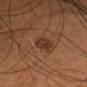Q: Was a biopsy performed?
A: total-body-photography surveillance lesion; no biopsy
Q: Patient demographics?
A: female, approximately 40 years of age
Q: What did automated image analysis measure?
A: a footprint of about 4.5 mm², an eccentricity of roughly 0.85, and a shape-asymmetry score of about 0.25 (0 = symmetric); an average lesion color of about L≈34 a*≈21 b*≈29 (CIELAB), about 9 CIELAB-L* units darker than the surrounding skin, and a normalized lesion–skin contrast near 8; border irregularity of about 2.5 on a 0–10 scale
Q: How large is the lesion?
A: ≈3 mm
Q: What is the imaging modality?
A: total-body-photography crop, ~15 mm field of view
Q: Lesion location?
A: the head or neck
Q: How was the tile lit?
A: cross-polarized